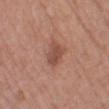follow-up = catalogued during a skin exam; not biopsied
lighting = white-light illumination
size = ~3 mm (longest diameter)
subject = female, aged 38–42
site = the left thigh
TBP lesion metrics = a symmetry-axis asymmetry near 0.25; a lesion color around L≈49 a*≈23 b*≈27 in CIELAB, about 9 CIELAB-L* units darker than the surrounding skin, and a normalized border contrast of about 7; a color-variation rating of about 2.5/10; a lesion-detection confidence of about 100/100
acquisition = ~15 mm crop, total-body skin-cancer survey